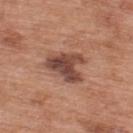Case summary:
– image · ~15 mm crop, total-body skin-cancer survey
– subject · male, aged 68–72
– body site · the back
– illumination · white-light illumination
– automated metrics · a lesion area of about 12 mm², an eccentricity of roughly 0.65, and a symmetry-axis asymmetry near 0.45; an average lesion color of about L≈46 a*≈22 b*≈26 (CIELAB), about 13 CIELAB-L* units darker than the surrounding skin, and a normalized border contrast of about 10; an automated nevus-likeness rating near 35 out of 100 and a lesion-detection confidence of about 100/100
– lesion diameter · ~4.5 mm (longest diameter)